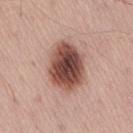Context: Automated tile analysis of the lesion measured an area of roughly 20 mm², an outline eccentricity of about 0.7 (0 = round, 1 = elongated), and two-axis asymmetry of about 0.1. The software also gave an average lesion color of about L≈48 a*≈23 b*≈25 (CIELAB) and a lesion–skin lightness drop of about 20. The analysis additionally found an automated nevus-likeness rating near 80 out of 100. The lesion is located on the lower back. The recorded lesion diameter is about 6 mm. A close-up tile cropped from a whole-body skin photograph, about 15 mm across. The patient is a male roughly 55 years of age.